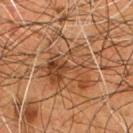Captured during whole-body skin photography for melanoma surveillance; the lesion was not biopsied. The lesion is on the chest. About 7.5 mm across. The subject is a male in their mid- to late 50s. A 15 mm crop from a total-body photograph taken for skin-cancer surveillance.